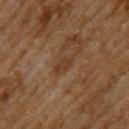workup: imaged on a skin check; not biopsied
body site: the upper back
imaging modality: ~15 mm crop, total-body skin-cancer survey
illumination: cross-polarized
lesion diameter: about 2.5 mm
patient: male, aged 63–67